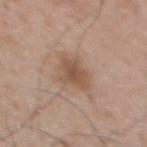<case>
  <biopsy_status>not biopsied; imaged during a skin examination</biopsy_status>
</case>